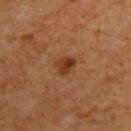image-analysis metrics=an area of roughly 4.5 mm² and a shape eccentricity near 0.6; roughly 10 lightness units darker than nearby skin; a color-variation rating of about 4/10 and peripheral color asymmetry of about 1.5; an automated nevus-likeness rating near 90 out of 100 and a detector confidence of about 100 out of 100 that the crop contains a lesion
subject=male, roughly 60 years of age
body site=the upper back
lighting=cross-polarized
acquisition=~15 mm tile from a whole-body skin photo
size=~2.5 mm (longest diameter)